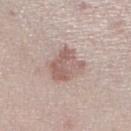Impression:
The lesion was tiled from a total-body skin photograph and was not biopsied.
Acquisition and patient details:
The lesion is located on the right lower leg. The patient is a male roughly 60 years of age. Cropped from a total-body skin-imaging series; the visible field is about 15 mm.